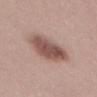Case summary:
* biopsy status: no biopsy performed (imaged during a skin exam)
* illumination: white-light illumination
* site: the abdomen
* patient: female, about 25 years old
* imaging modality: total-body-photography crop, ~15 mm field of view